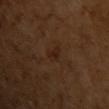Imaged during a routine full-body skin examination; the lesion was not biopsied and no histopathology is available. The lesion is on the chest. The patient is a female approximately 55 years of age. About 3 mm across. A 15 mm crop from a total-body photograph taken for skin-cancer surveillance.